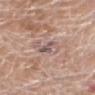Image and clinical context: Measured at roughly 3 mm in maximum diameter. A 15 mm close-up extracted from a 3D total-body photography capture. The lesion is located on the head or neck. This is a white-light tile. A male patient, roughly 55 years of age.